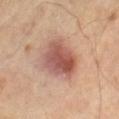Imaged during a routine full-body skin examination; the lesion was not biopsied and no histopathology is available. Located on the right thigh. A male patient about 70 years old. A lesion tile, about 15 mm wide, cut from a 3D total-body photograph.